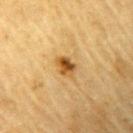Assessment:
Captured during whole-body skin photography for melanoma surveillance; the lesion was not biopsied.
Image and clinical context:
A male patient, aged 83 or over. A 15 mm crop from a total-body photograph taken for skin-cancer surveillance. Located on the left upper arm.